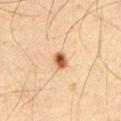<tbp_lesion>
  <biopsy_status>not biopsied; imaged during a skin examination</biopsy_status>
  <image>
    <source>total-body photography crop</source>
    <field_of_view_mm>15</field_of_view_mm>
  </image>
  <lighting>cross-polarized</lighting>
  <lesion_size>
    <long_diameter_mm_approx>2.0</long_diameter_mm_approx>
  </lesion_size>
  <site>abdomen</site>
  <patient>
    <sex>male</sex>
    <age_approx>60</age_approx>
  </patient>
</tbp_lesion>